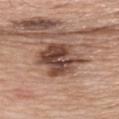This lesion was catalogued during total-body skin photography and was not selected for biopsy. Measured at roughly 6.5 mm in maximum diameter. A male patient, aged 78–82. Automated image analysis of the tile measured a lesion area of about 19 mm², an eccentricity of roughly 0.75, and a symmetry-axis asymmetry near 0.2. The software also gave a border-irregularity rating of about 3/10 and a peripheral color-asymmetry measure near 3. It also reported a nevus-likeness score of about 5/100 and lesion-presence confidence of about 100/100. Located on the chest. A roughly 15 mm field-of-view crop from a total-body skin photograph. Imaged with white-light lighting.